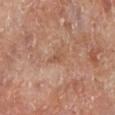biopsy_status: not biopsied; imaged during a skin examination
image:
  source: total-body photography crop
  field_of_view_mm: 15
lesion_size:
  long_diameter_mm_approx: 2.5
patient:
  sex: male
  age_approx: 65
site: leg
lighting: cross-polarized